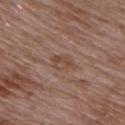Q: Is there a histopathology result?
A: catalogued during a skin exam; not biopsied
Q: What are the patient's age and sex?
A: male, about 55 years old
Q: Where on the body is the lesion?
A: the upper back
Q: How large is the lesion?
A: ~3 mm (longest diameter)
Q: What kind of image is this?
A: ~15 mm crop, total-body skin-cancer survey
Q: How was the tile lit?
A: white-light illumination